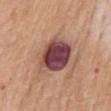| feature | finding |
|---|---|
| workup | total-body-photography surveillance lesion; no biopsy |
| illumination | white-light illumination |
| site | the mid back |
| TBP lesion metrics | a symmetry-axis asymmetry near 0.15; a mean CIELAB color near L≈45 a*≈24 b*≈20 and a lesion–skin lightness drop of about 18 |
| diameter | ~4.5 mm (longest diameter) |
| imaging modality | ~15 mm tile from a whole-body skin photo |
| subject | male, roughly 65 years of age |